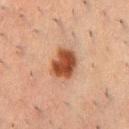The lesion was tiled from a total-body skin photograph and was not biopsied. The recorded lesion diameter is about 4 mm. Located on the chest. A male subject, approximately 50 years of age. A lesion tile, about 15 mm wide, cut from a 3D total-body photograph. Captured under cross-polarized illumination.